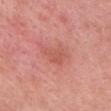Captured during whole-body skin photography for melanoma surveillance; the lesion was not biopsied. Cropped from a total-body skin-imaging series; the visible field is about 15 mm. The total-body-photography lesion software estimated a lesion area of about 6 mm², an outline eccentricity of about 0.75 (0 = round, 1 = elongated), and two-axis asymmetry of about 0.45. It also reported a border-irregularity index near 4.5/10, internal color variation of about 1.5 on a 0–10 scale, and a peripheral color-asymmetry measure near 0.5. A female subject, about 50 years old. The lesion is on the head or neck. Longest diameter approximately 3.5 mm. Imaged with white-light lighting.